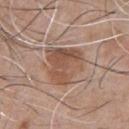| key | value |
|---|---|
| body site | the chest |
| tile lighting | white-light |
| TBP lesion metrics | a border-irregularity rating of about 4/10, internal color variation of about 5 on a 0–10 scale, and radial color variation of about 1.5 |
| imaging modality | 15 mm crop, total-body photography |
| patient | male, roughly 45 years of age |